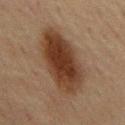The lesion was photographed on a routine skin check and not biopsied; there is no pathology result. The lesion's longest dimension is about 9 mm. A male patient about 70 years old. A 15 mm close-up extracted from a 3D total-body photography capture. The tile uses cross-polarized illumination. From the mid back.